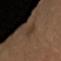biopsy status: no biopsy performed (imaged during a skin exam) | subject: male, in their mid- to late 40s | diameter: ≈3 mm | acquisition: ~15 mm crop, total-body skin-cancer survey | body site: the right forearm.